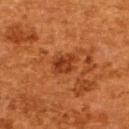<case>
  <biopsy_status>not biopsied; imaged during a skin examination</biopsy_status>
  <image>
    <source>total-body photography crop</source>
    <field_of_view_mm>15</field_of_view_mm>
  </image>
  <site>upper back</site>
  <lighting>cross-polarized</lighting>
  <automated_metrics>
    <area_mm2_approx>5.5</area_mm2_approx>
    <eccentricity>0.65</eccentricity>
    <shape_asymmetry>0.3</shape_asymmetry>
    <nevus_likeness_0_100>85</nevus_likeness_0_100>
    <lesion_detection_confidence_0_100>100</lesion_detection_confidence_0_100>
  </automated_metrics>
  <patient>
    <sex>female</sex>
    <age_approx>50</age_approx>
  </patient>
</case>